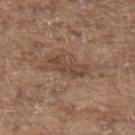No biopsy was performed on this lesion — it was imaged during a full skin examination and was not determined to be concerning. Automated tile analysis of the lesion measured about 9 CIELAB-L* units darker than the surrounding skin. The analysis additionally found a border-irregularity index near 4.5/10, a color-variation rating of about 2.5/10, and radial color variation of about 1. The analysis additionally found a nevus-likeness score of about 0/100 and a lesion-detection confidence of about 55/100. Located on the back. A 15 mm crop from a total-body photograph taken for skin-cancer surveillance. This is a white-light tile. The lesion's longest dimension is about 5 mm. A male subject, roughly 80 years of age.